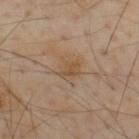Assessment:
This lesion was catalogued during total-body skin photography and was not selected for biopsy.
Background:
Cropped from a total-body skin-imaging series; the visible field is about 15 mm. The subject is a male about 55 years old. The total-body-photography lesion software estimated a footprint of about 5 mm² and two-axis asymmetry of about 0.25. It also reported a border-irregularity rating of about 2.5/10, a within-lesion color-variation index near 3/10, and a peripheral color-asymmetry measure near 1. The software also gave a nevus-likeness score of about 5/100 and lesion-presence confidence of about 100/100. The lesion is on the mid back.